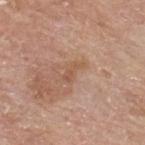Q: Was this lesion biopsied?
A: catalogued during a skin exam; not biopsied
Q: How large is the lesion?
A: about 3 mm
Q: Patient demographics?
A: male, approximately 80 years of age
Q: What did automated image analysis measure?
A: an eccentricity of roughly 0.9 and a symmetry-axis asymmetry near 0.4; an average lesion color of about L≈55 a*≈20 b*≈31 (CIELAB) and a normalized lesion–skin contrast near 5.5
Q: What lighting was used for the tile?
A: white-light illumination
Q: Where on the body is the lesion?
A: the upper back
Q: What is the imaging modality?
A: total-body-photography crop, ~15 mm field of view